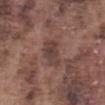The lesion was photographed on a routine skin check and not biopsied; there is no pathology result. Captured under white-light illumination. A 15 mm close-up tile from a total-body photography series done for melanoma screening. Located on the abdomen. A male subject, roughly 75 years of age. Automated image analysis of the tile measured an eccentricity of roughly 0.85 and a shape-asymmetry score of about 0.25 (0 = symmetric). And it measured an average lesion color of about L≈40 a*≈18 b*≈21 (CIELAB) and roughly 9 lightness units darker than nearby skin. The analysis additionally found a nevus-likeness score of about 0/100 and a lesion-detection confidence of about 95/100.